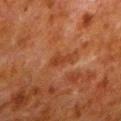Findings:
– biopsy status — catalogued during a skin exam; not biopsied
– automated metrics — a mean CIELAB color near L≈32 a*≈23 b*≈30, a lesion–skin lightness drop of about 6, and a normalized border contrast of about 6; internal color variation of about 0.5 on a 0–10 scale and radial color variation of about 0; a classifier nevus-likeness of about 0/100 and a detector confidence of about 100 out of 100 that the crop contains a lesion
– image source — ~15 mm crop, total-body skin-cancer survey
– size — ≈2.5 mm
– patient — male, in their 80s
– body site — the left lower leg